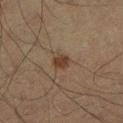Q: Who is the patient?
A: male, in their 60s
Q: What did automated image analysis measure?
A: a shape eccentricity near 0.6 and a symmetry-axis asymmetry near 0.25; a nevus-likeness score of about 85/100 and a lesion-detection confidence of about 100/100
Q: What kind of image is this?
A: total-body-photography crop, ~15 mm field of view
Q: How was the tile lit?
A: cross-polarized
Q: Lesion location?
A: the left lower leg
Q: Lesion size?
A: ≈2 mm